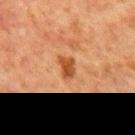Captured during whole-body skin photography for melanoma surveillance; the lesion was not biopsied.